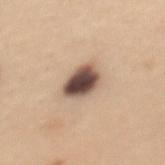No biopsy was performed on this lesion — it was imaged during a full skin examination and was not determined to be concerning.
A 15 mm crop from a total-body photograph taken for skin-cancer surveillance.
A female subject aged around 30.
The lesion is on the mid back.